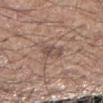A 15 mm close-up tile from a total-body photography series done for melanoma screening. A male patient aged around 65. An algorithmic analysis of the crop reported a shape eccentricity near 0.4 and two-axis asymmetry of about 0.25. The software also gave a lesion color around L≈50 a*≈16 b*≈23 in CIELAB, roughly 8 lightness units darker than nearby skin, and a lesion-to-skin contrast of about 6.5 (normalized; higher = more distinct). Imaged with white-light lighting. Located on the right forearm.